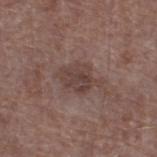The lesion is located on the right lower leg. This is a white-light tile. The total-body-photography lesion software estimated a footprint of about 9 mm², a shape eccentricity near 0.7, and a symmetry-axis asymmetry near 0.25. And it measured a classifier nevus-likeness of about 0/100. A male patient, approximately 70 years of age. A 15 mm close-up tile from a total-body photography series done for melanoma screening.